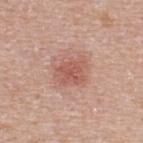Captured during whole-body skin photography for melanoma surveillance; the lesion was not biopsied.
The total-body-photography lesion software estimated a lesion area of about 10 mm², an outline eccentricity of about 0.55 (0 = round, 1 = elongated), and a shape-asymmetry score of about 0.2 (0 = symmetric). The software also gave a border-irregularity index near 2.5/10, a color-variation rating of about 3/10, and peripheral color asymmetry of about 1.
The patient is a female aged around 40.
Cropped from a whole-body photographic skin survey; the tile spans about 15 mm.
On the upper back.
The recorded lesion diameter is about 4 mm.